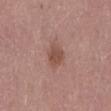{
  "biopsy_status": "not biopsied; imaged during a skin examination",
  "patient": {
    "sex": "female",
    "age_approx": 35
  },
  "automated_metrics": {
    "area_mm2_approx": 5.0,
    "eccentricity": 0.6,
    "shape_asymmetry": 0.25,
    "cielab_L": 49,
    "cielab_a": 22,
    "cielab_b": 25,
    "vs_skin_darker_L": 9.0,
    "vs_skin_contrast_norm": 7.0,
    "border_irregularity_0_10": 2.0,
    "color_variation_0_10": 2.5,
    "peripheral_color_asymmetry": 1.0,
    "lesion_detection_confidence_0_100": 100
  },
  "lighting": "white-light",
  "lesion_size": {
    "long_diameter_mm_approx": 2.5
  },
  "image": {
    "source": "total-body photography crop",
    "field_of_view_mm": 15
  },
  "site": "lower back"
}